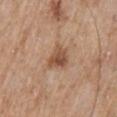A male patient, approximately 60 years of age. This is a white-light tile. This image is a 15 mm lesion crop taken from a total-body photograph. The recorded lesion diameter is about 3 mm. Automated image analysis of the tile measured a nevus-likeness score of about 60/100 and lesion-presence confidence of about 100/100. The lesion is on the mid back.